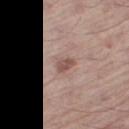| feature | finding |
|---|---|
| notes | imaged on a skin check; not biopsied |
| illumination | white-light illumination |
| site | the right thigh |
| image | total-body-photography crop, ~15 mm field of view |
| TBP lesion metrics | about 11 CIELAB-L* units darker than the surrounding skin; border irregularity of about 2.5 on a 0–10 scale |
| size | ~2.5 mm (longest diameter) |
| subject | male, approximately 55 years of age |